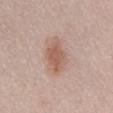{
  "biopsy_status": "not biopsied; imaged during a skin examination"
}